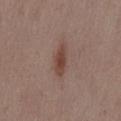biopsy_status: not biopsied; imaged during a skin examination
lighting: white-light
patient:
  sex: female
  age_approx: 50
image:
  source: total-body photography crop
  field_of_view_mm: 15
automated_metrics:
  area_mm2_approx: 5.0
  eccentricity: 0.9
  shape_asymmetry: 0.2
lesion_size:
  long_diameter_mm_approx: 4.0
site: back